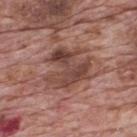The lesion was tiled from a total-body skin photograph and was not biopsied.
A region of skin cropped from a whole-body photographic capture, roughly 15 mm wide.
The lesion is located on the upper back.
The patient is a male roughly 70 years of age.
Automated tile analysis of the lesion measured a border-irregularity rating of about 6.5/10, a color-variation rating of about 6/10, and radial color variation of about 2. The analysis additionally found an automated nevus-likeness rating near 5 out of 100.
Measured at roughly 6.5 mm in maximum diameter.
Imaged with white-light lighting.